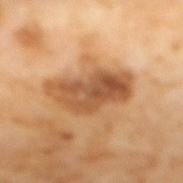Q: Was a biopsy performed?
A: catalogued during a skin exam; not biopsied
Q: How large is the lesion?
A: about 7 mm
Q: Who is the patient?
A: female, aged 53 to 57
Q: Where on the body is the lesion?
A: the mid back
Q: How was this image acquired?
A: total-body-photography crop, ~15 mm field of view
Q: Illumination type?
A: cross-polarized illumination